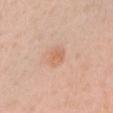| feature | finding |
|---|---|
| workup | total-body-photography surveillance lesion; no biopsy |
| automated lesion analysis | a footprint of about 4.5 mm² and a shape-asymmetry score of about 0.2 (0 = symmetric); an average lesion color of about L≈65 a*≈22 b*≈33 (CIELAB) and about 7 CIELAB-L* units darker than the surrounding skin |
| body site | the head or neck |
| imaging modality | ~15 mm tile from a whole-body skin photo |
| lesion diameter | ~2.5 mm (longest diameter) |
| patient | female, approximately 20 years of age |